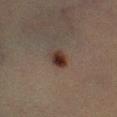This lesion was catalogued during total-body skin photography and was not selected for biopsy. Located on the left lower leg. Automated image analysis of the tile measured a mean CIELAB color near L≈26 a*≈14 b*≈19 and a normalized border contrast of about 10.5. The subject is a male about 40 years old. The tile uses cross-polarized illumination. Approximately 3 mm at its widest. A 15 mm close-up tile from a total-body photography series done for melanoma screening.